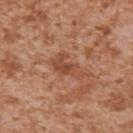Q: Is there a histopathology result?
A: catalogued during a skin exam; not biopsied
Q: What are the patient's age and sex?
A: male, in their mid- to late 40s
Q: What is the imaging modality?
A: ~15 mm crop, total-body skin-cancer survey
Q: Where on the body is the lesion?
A: the right upper arm